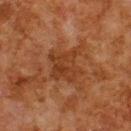Findings:
* notes: no biopsy performed (imaged during a skin exam)
* tile lighting: cross-polarized
* image: 15 mm crop, total-body photography
* location: the upper back
* automated lesion analysis: a footprint of about 12 mm² and a shape-asymmetry score of about 0.3 (0 = symmetric); an automated nevus-likeness rating near 0 out of 100 and lesion-presence confidence of about 100/100
* subject: male, aged 78 to 82
* lesion diameter: ~4.5 mm (longest diameter)